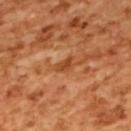workup = total-body-photography surveillance lesion; no biopsy | illumination = cross-polarized illumination | patient = female, in their mid- to late 50s | location = the upper back | acquisition = ~15 mm tile from a whole-body skin photo.